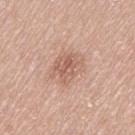Q: Is there a histopathology result?
A: no biopsy performed (imaged during a skin exam)
Q: What is the lesion's diameter?
A: about 4 mm
Q: Where on the body is the lesion?
A: the left thigh
Q: Automated lesion metrics?
A: a border-irregularity index near 3/10 and internal color variation of about 4 on a 0–10 scale
Q: How was this image acquired?
A: 15 mm crop, total-body photography
Q: Patient demographics?
A: male, roughly 75 years of age